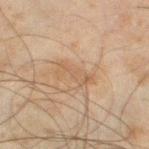Findings:
– biopsy status: no biopsy performed (imaged during a skin exam)
– site: the left thigh
– size: ≈4 mm
– patient: male, aged approximately 45
– illumination: cross-polarized
– image source: 15 mm crop, total-body photography